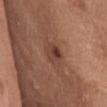This is a white-light tile. Measured at roughly 3 mm in maximum diameter. The lesion-visualizer software estimated an area of roughly 5.5 mm², an outline eccentricity of about 0.65 (0 = round, 1 = elongated), and two-axis asymmetry of about 0.15. The software also gave an average lesion color of about L≈41 a*≈22 b*≈27 (CIELAB). This image is a 15 mm lesion crop taken from a total-body photograph. A female patient aged 63 to 67. The lesion is located on the abdomen.